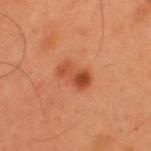Q: Is there a histopathology result?
A: no biopsy performed (imaged during a skin exam)
Q: Where on the body is the lesion?
A: the upper back
Q: What is the lesion's diameter?
A: about 3.5 mm
Q: What are the patient's age and sex?
A: male, about 55 years old
Q: What is the imaging modality?
A: ~15 mm tile from a whole-body skin photo